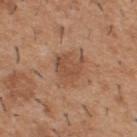Assessment:
The lesion was photographed on a routine skin check and not biopsied; there is no pathology result.